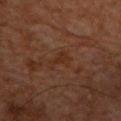Q: Is there a histopathology result?
A: total-body-photography surveillance lesion; no biopsy
Q: What are the patient's age and sex?
A: male, approximately 60 years of age
Q: How was this image acquired?
A: total-body-photography crop, ~15 mm field of view
Q: How large is the lesion?
A: ≈2.5 mm
Q: Illumination type?
A: cross-polarized
Q: Lesion location?
A: the back